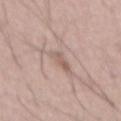Case summary:
- follow-up: total-body-photography surveillance lesion; no biopsy
- image source: 15 mm crop, total-body photography
- size: ≈3.5 mm
- patient: male, about 55 years old
- TBP lesion metrics: an eccentricity of roughly 0.9 and two-axis asymmetry of about 0.3; a color-variation rating of about 2/10 and peripheral color asymmetry of about 0.5
- tile lighting: white-light